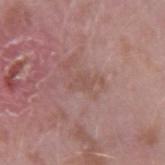Captured during whole-body skin photography for melanoma surveillance; the lesion was not biopsied. A male subject in their 40s. Automated image analysis of the tile measured a lesion area of about 3 mm², a shape eccentricity near 0.8, and a symmetry-axis asymmetry near 0.4. And it measured an average lesion color of about L≈51 a*≈20 b*≈24 (CIELAB) and a normalized lesion–skin contrast near 4.5. It also reported an automated nevus-likeness rating near 0 out of 100 and a detector confidence of about 100 out of 100 that the crop contains a lesion. This image is a 15 mm lesion crop taken from a total-body photograph. Imaged with white-light lighting. On the right upper arm. Approximately 2.5 mm at its widest.